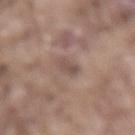  biopsy_status: not biopsied; imaged during a skin examination
  lesion_size:
    long_diameter_mm_approx: 3.5
  image:
    source: total-body photography crop
    field_of_view_mm: 15
  site: lower back
  patient:
    sex: male
    age_approx: 75
  lighting: white-light